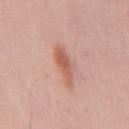Case summary:
– biopsy status · no biopsy performed (imaged during a skin exam)
– lesion size · ~4.5 mm (longest diameter)
– body site · the back
– lighting · white-light illumination
– patient · male, aged 38 to 42
– image source · 15 mm crop, total-body photography